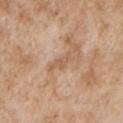Background: The patient is a male in their mid- to late 60s. About 3.5 mm across. Located on the arm. Cropped from a whole-body photographic skin survey; the tile spans about 15 mm. Imaged with white-light lighting. The total-body-photography lesion software estimated border irregularity of about 4.5 on a 0–10 scale, internal color variation of about 2 on a 0–10 scale, and peripheral color asymmetry of about 1. It also reported a classifier nevus-likeness of about 0/100 and a lesion-detection confidence of about 100/100.